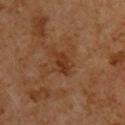workup=imaged on a skin check; not biopsied | subject=male, aged around 60 | image source=15 mm crop, total-body photography | lesion diameter=about 3 mm | site=the upper back | illumination=cross-polarized | automated lesion analysis=a lesion color around L≈34 a*≈23 b*≈32 in CIELAB, roughly 7 lightness units darker than nearby skin, and a normalized lesion–skin contrast near 7; a within-lesion color-variation index near 3/10 and a peripheral color-asymmetry measure near 1; a nevus-likeness score of about 5/100 and a lesion-detection confidence of about 100/100.